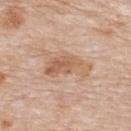  biopsy_status: not biopsied; imaged during a skin examination
  lesion_size:
    long_diameter_mm_approx: 6.0
  image:
    source: total-body photography crop
    field_of_view_mm: 15
  automated_metrics:
    area_mm2_approx: 14.0
    eccentricity: 0.85
    shape_asymmetry: 0.4
  lighting: white-light
  site: upper back
  patient:
    sex: male
    age_approx: 85+